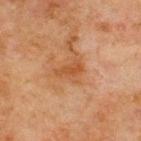{
  "biopsy_status": "not biopsied; imaged during a skin examination",
  "lesion_size": {
    "long_diameter_mm_approx": 3.5
  },
  "automated_metrics": {
    "nevus_likeness_0_100": 0,
    "lesion_detection_confidence_0_100": 100
  },
  "site": "back",
  "image": {
    "source": "total-body photography crop",
    "field_of_view_mm": 15
  },
  "patient": {
    "sex": "male",
    "age_approx": 70
  },
  "lighting": "cross-polarized"
}